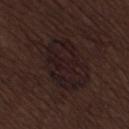subject: male, approximately 70 years of age; tile lighting: white-light illumination; lesion diameter: ~7 mm (longest diameter); site: the back; acquisition: ~15 mm tile from a whole-body skin photo.